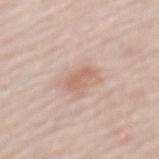| key | value |
|---|---|
| workup | total-body-photography surveillance lesion; no biopsy |
| TBP lesion metrics | a lesion area of about 4 mm² and an outline eccentricity of about 0.85 (0 = round, 1 = elongated); a lesion color around L≈63 a*≈20 b*≈28 in CIELAB, about 9 CIELAB-L* units darker than the surrounding skin, and a normalized border contrast of about 6 |
| lighting | white-light illumination |
| patient | female, in their mid-60s |
| image source | 15 mm crop, total-body photography |
| anatomic site | the mid back |
| lesion size | ≈3 mm |